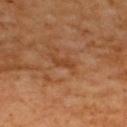follow-up=total-body-photography surveillance lesion; no biopsy
subject=male, aged 58–62
lesion size=≈3 mm
image-analysis metrics=a mean CIELAB color near L≈42 a*≈24 b*≈36, about 7 CIELAB-L* units darker than the surrounding skin, and a normalized lesion–skin contrast near 6; a peripheral color-asymmetry measure near 0; an automated nevus-likeness rating near 0 out of 100 and a detector confidence of about 100 out of 100 that the crop contains a lesion
body site=the upper back
image source=~15 mm tile from a whole-body skin photo
illumination=cross-polarized